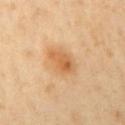The lesion was photographed on a routine skin check and not biopsied; there is no pathology result.
The recorded lesion diameter is about 3.5 mm.
A female patient aged 48–52.
From the arm.
The tile uses cross-polarized illumination.
This image is a 15 mm lesion crop taken from a total-body photograph.